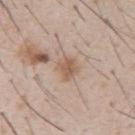The lesion was tiled from a total-body skin photograph and was not biopsied. Approximately 3 mm at its widest. A 15 mm close-up tile from a total-body photography series done for melanoma screening. Automated tile analysis of the lesion measured a mean CIELAB color near L≈58 a*≈17 b*≈29, a lesion–skin lightness drop of about 9, and a lesion-to-skin contrast of about 7 (normalized; higher = more distinct). A male patient in their mid-50s.